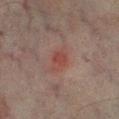imaging modality: 15 mm crop, total-body photography
size: about 3.5 mm
subject: male, in their mid-70s
body site: the left lower leg